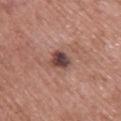Background:
A female patient aged 58–62. The lesion is on the upper back. Cropped from a whole-body photographic skin survey; the tile spans about 15 mm. This is a white-light tile. Measured at roughly 2.5 mm in maximum diameter.